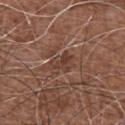notes = catalogued during a skin exam; not biopsied | TBP lesion metrics = an area of roughly 5 mm² and an eccentricity of roughly 0.7; an average lesion color of about L≈39 a*≈20 b*≈25 (CIELAB), a lesion–skin lightness drop of about 7, and a normalized lesion–skin contrast near 6 | tile lighting = white-light | subject = male, aged 73 to 77 | imaging modality = total-body-photography crop, ~15 mm field of view | size = ~3 mm (longest diameter) | anatomic site = the chest.